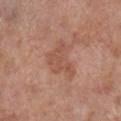Q: How was this image acquired?
A: ~15 mm crop, total-body skin-cancer survey
Q: What are the patient's age and sex?
A: male, aged 68–72
Q: What is the anatomic site?
A: the right lower leg
Q: How was the tile lit?
A: white-light illumination